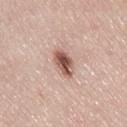Q: Is there a histopathology result?
A: total-body-photography surveillance lesion; no biopsy
Q: Lesion location?
A: the right thigh
Q: What did automated image analysis measure?
A: a lesion area of about 6 mm² and an eccentricity of roughly 0.85; a border-irregularity rating of about 2/10, a color-variation rating of about 6/10, and radial color variation of about 2; an automated nevus-likeness rating near 95 out of 100
Q: What lighting was used for the tile?
A: white-light illumination
Q: Patient demographics?
A: female, aged 48 to 52
Q: What kind of image is this?
A: total-body-photography crop, ~15 mm field of view